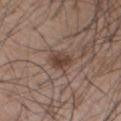Imaged during a routine full-body skin examination; the lesion was not biopsied and no histopathology is available. The subject is a male roughly 55 years of age. Imaged with white-light lighting. Located on the chest. Approximately 3.5 mm at its widest. A 15 mm crop from a total-body photograph taken for skin-cancer surveillance. The total-body-photography lesion software estimated a lesion area of about 5 mm² and an eccentricity of roughly 0.85. The software also gave a border-irregularity rating of about 2.5/10, internal color variation of about 2 on a 0–10 scale, and a peripheral color-asymmetry measure near 1.